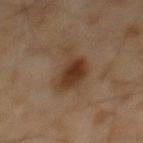This lesion was catalogued during total-body skin photography and was not selected for biopsy.
Cropped from a whole-body photographic skin survey; the tile spans about 15 mm.
Automated tile analysis of the lesion measured an average lesion color of about L≈33 a*≈16 b*≈27 (CIELAB). The software also gave a within-lesion color-variation index near 4/10 and radial color variation of about 1.
Located on the chest.
Approximately 5.5 mm at its widest.
A male subject aged 58 to 62.
Captured under cross-polarized illumination.